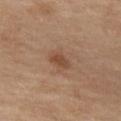<lesion>
<biopsy_status>not biopsied; imaged during a skin examination</biopsy_status>
<lesion_size>
  <long_diameter_mm_approx>2.5</long_diameter_mm_approx>
</lesion_size>
<patient>
  <sex>female</sex>
  <age_approx>55</age_approx>
</patient>
<lighting>cross-polarized</lighting>
<site>left thigh</site>
<image>
  <source>total-body photography crop</source>
  <field_of_view_mm>15</field_of_view_mm>
</image>
<automated_metrics>
  <area_mm2_approx>4.0</area_mm2_approx>
  <eccentricity>0.75</eccentricity>
  <shape_asymmetry>0.3</shape_asymmetry>
  <cielab_L>47</cielab_L>
  <cielab_a>20</cielab_a>
  <cielab_b>31</cielab_b>
  <vs_skin_darker_L>8.0</vs_skin_darker_L>
  <vs_skin_contrast_norm>7.0</vs_skin_contrast_norm>
  <border_irregularity_0_10>2.5</border_irregularity_0_10>
  <peripheral_color_asymmetry>1.0</peripheral_color_asymmetry>
</automated_metrics>
</lesion>